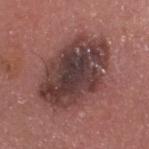workup: total-body-photography surveillance lesion; no biopsy | patient: male, aged approximately 55 | lighting: white-light illumination | image: ~15 mm tile from a whole-body skin photo | diameter: about 9 mm | anatomic site: the head or neck.